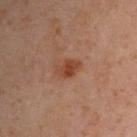Impression: Part of a total-body skin-imaging series; this lesion was reviewed on a skin check and was not flagged for biopsy. Acquisition and patient details: A 15 mm close-up extracted from a 3D total-body photography capture. A male patient about 50 years old. This is a cross-polarized tile. Longest diameter approximately 3 mm. From the left upper arm. Automated image analysis of the tile measured a lesion area of about 4.5 mm², an outline eccentricity of about 0.7 (0 = round, 1 = elongated), and two-axis asymmetry of about 0.25. The analysis additionally found about 8 CIELAB-L* units darker than the surrounding skin.